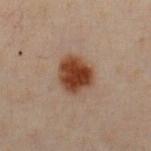notes: total-body-photography surveillance lesion; no biopsy
lighting: cross-polarized illumination
size: ≈4 mm
anatomic site: the chest
subject: male, approximately 50 years of age
image source: total-body-photography crop, ~15 mm field of view
automated lesion analysis: a lesion area of about 11 mm² and an eccentricity of roughly 0.45; a border-irregularity index near 1.5/10, a color-variation rating of about 4/10, and radial color variation of about 1